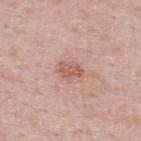Part of a total-body skin-imaging series; this lesion was reviewed on a skin check and was not flagged for biopsy. A 15 mm crop from a total-body photograph taken for skin-cancer surveillance. The lesion is on the upper back. The subject is a male approximately 50 years of age. This is a white-light tile. Approximately 3 mm at its widest.